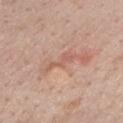biopsy_status: not biopsied; imaged during a skin examination
image:
  source: total-body photography crop
  field_of_view_mm: 15
lesion_size:
  long_diameter_mm_approx: 3.5
patient:
  sex: female
  age_approx: 50
site: chest
automated_metrics:
  cielab_L: 59
  cielab_a: 23
  cielab_b: 29
  vs_skin_darker_L: 7.0
  vs_skin_contrast_norm: 5.0
  nevus_likeness_0_100: 0
lighting: white-light